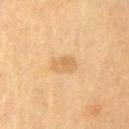follow-up — catalogued during a skin exam; not biopsied | patient — male, aged around 85 or older | image source — ~15 mm crop, total-body skin-cancer survey | body site — the left upper arm | illumination — cross-polarized illumination | lesion size — ≈3 mm.